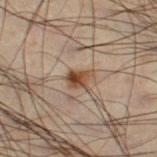Assessment: Recorded during total-body skin imaging; not selected for excision or biopsy. Background: About 2.5 mm across. From the right thigh. This image is a 15 mm lesion crop taken from a total-body photograph. A male subject aged around 50.